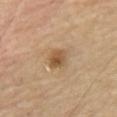Impression:
Recorded during total-body skin imaging; not selected for excision or biopsy.
Context:
Cropped from a total-body skin-imaging series; the visible field is about 15 mm. A male patient, aged 68 to 72. From the chest.